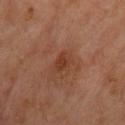The lesion was photographed on a routine skin check and not biopsied; there is no pathology result. From the front of the torso. The subject is a male about 65 years old. This image is a 15 mm lesion crop taken from a total-body photograph.